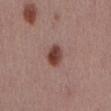| key | value |
|---|---|
| biopsy status | total-body-photography surveillance lesion; no biopsy |